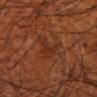biopsy status: catalogued during a skin exam; not biopsied
anatomic site: the left forearm
patient: male, roughly 70 years of age
image source: ~15 mm crop, total-body skin-cancer survey
size: ≈4.5 mm
lighting: cross-polarized